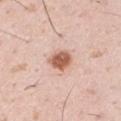This lesion was catalogued during total-body skin photography and was not selected for biopsy. A male subject approximately 35 years of age. This is a white-light tile. A 15 mm close-up extracted from a 3D total-body photography capture. The total-body-photography lesion software estimated a lesion area of about 6 mm², a shape eccentricity near 0.5, and a shape-asymmetry score of about 0.2 (0 = symmetric). And it measured an average lesion color of about L≈60 a*≈24 b*≈30 (CIELAB) and a lesion-to-skin contrast of about 10.5 (normalized; higher = more distinct). Located on the right upper arm.